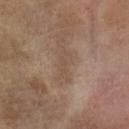The lesion was tiled from a total-body skin photograph and was not biopsied.
Imaged with cross-polarized lighting.
The total-body-photography lesion software estimated a footprint of about 3.5 mm² and an eccentricity of roughly 0.95.
The lesion is on the left forearm.
This image is a 15 mm lesion crop taken from a total-body photograph.
The patient is a female aged 73 to 77.
The lesion's longest dimension is about 3.5 mm.